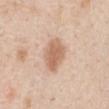diameter: ≈4 mm | illumination: white-light illumination | subject: male, aged approximately 60 | body site: the front of the torso | image source: ~15 mm tile from a whole-body skin photo.